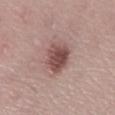The lesion was photographed on a routine skin check and not biopsied; there is no pathology result.
The subject is a female about 40 years old.
On the right lower leg.
A 15 mm close-up tile from a total-body photography series done for melanoma screening.
Imaged with white-light lighting.
The recorded lesion diameter is about 4 mm.
The total-body-photography lesion software estimated a mean CIELAB color near L≈48 a*≈21 b*≈21, roughly 14 lightness units darker than nearby skin, and a lesion-to-skin contrast of about 9.5 (normalized; higher = more distinct). And it measured a border-irregularity index near 2/10 and internal color variation of about 4.5 on a 0–10 scale. The analysis additionally found a nevus-likeness score of about 90/100 and a detector confidence of about 100 out of 100 that the crop contains a lesion.